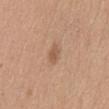follow-up = total-body-photography surveillance lesion; no biopsy | image-analysis metrics = a lesion area of about 3 mm²; a mean CIELAB color near L≈56 a*≈20 b*≈32, a lesion–skin lightness drop of about 9, and a normalized lesion–skin contrast near 6; border irregularity of about 2.5 on a 0–10 scale, a within-lesion color-variation index near 1/10, and a peripheral color-asymmetry measure near 0.5 | patient = female, about 50 years old | lesion size = about 2.5 mm | body site = the abdomen | image source = ~15 mm crop, total-body skin-cancer survey.